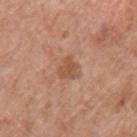Recorded during total-body skin imaging; not selected for excision or biopsy.
Captured under white-light illumination.
A 15 mm close-up tile from a total-body photography series done for melanoma screening.
The lesion is located on the left upper arm.
The patient is a female in their 60s.
An algorithmic analysis of the crop reported a lesion area of about 4.5 mm², an outline eccentricity of about 0.4 (0 = round, 1 = elongated), and a symmetry-axis asymmetry near 0.4. The analysis additionally found an average lesion color of about L≈53 a*≈23 b*≈32 (CIELAB), about 8 CIELAB-L* units darker than the surrounding skin, and a normalized lesion–skin contrast near 6.5. The analysis additionally found a border-irregularity index near 4/10. And it measured an automated nevus-likeness rating near 5 out of 100 and lesion-presence confidence of about 100/100.